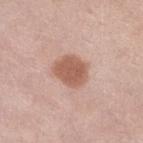• acquisition — total-body-photography crop, ~15 mm field of view
• subject — female, in their mid- to late 60s
• body site — the left leg